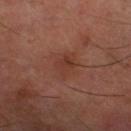| feature | finding |
|---|---|
| notes | total-body-photography surveillance lesion; no biopsy |
| subject | male, approximately 60 years of age |
| diameter | ~3.5 mm (longest diameter) |
| tile lighting | cross-polarized illumination |
| body site | the left lower leg |
| image source | ~15 mm crop, total-body skin-cancer survey |